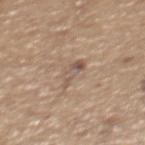Impression: The lesion was photographed on a routine skin check and not biopsied; there is no pathology result. Context: A male patient aged 63–67. This image is a 15 mm lesion crop taken from a total-body photograph. Located on the mid back. The tile uses white-light illumination. Approximately 3 mm at its widest.